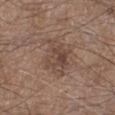Recorded during total-body skin imaging; not selected for excision or biopsy.
Automated image analysis of the tile measured a footprint of about 11 mm², an eccentricity of roughly 0.6, and a shape-asymmetry score of about 0.25 (0 = symmetric). The analysis additionally found a mean CIELAB color near L≈45 a*≈17 b*≈24, about 8 CIELAB-L* units darker than the surrounding skin, and a normalized lesion–skin contrast near 6. The software also gave a classifier nevus-likeness of about 5/100.
Imaged with white-light lighting.
Cropped from a total-body skin-imaging series; the visible field is about 15 mm.
The subject is a male aged 58–62.
The recorded lesion diameter is about 4.5 mm.
From the left lower leg.